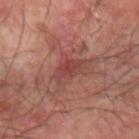Q: Was this lesion biopsied?
A: catalogued during a skin exam; not biopsied
Q: Who is the patient?
A: male, approximately 65 years of age
Q: How was this image acquired?
A: 15 mm crop, total-body photography
Q: Where on the body is the lesion?
A: the left forearm
Q: Illumination type?
A: cross-polarized illumination
Q: Lesion size?
A: ≈4.5 mm
Q: Automated lesion metrics?
A: a nevus-likeness score of about 0/100